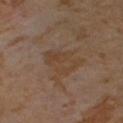The lesion was photographed on a routine skin check and not biopsied; there is no pathology result. This image is a 15 mm lesion crop taken from a total-body photograph. The subject is a female aged 53–57. Imaged with cross-polarized lighting. Located on the chest.